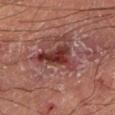Imaged during a routine full-body skin examination; the lesion was not biopsied and no histopathology is available. Longest diameter approximately 5 mm. A 15 mm crop from a total-body photograph taken for skin-cancer surveillance. The tile uses cross-polarized illumination. The lesion is on the leg. A male patient, approximately 55 years of age.